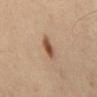  biopsy_status: not biopsied; imaged during a skin examination
  image:
    source: total-body photography crop
    field_of_view_mm: 15
  automated_metrics:
    area_mm2_approx: 4.5
    eccentricity: 0.85
    shape_asymmetry: 0.25
    cielab_L: 50
    cielab_a: 19
    cielab_b: 31
    vs_skin_contrast_norm: 9.5
    nevus_likeness_0_100: 100
  patient:
    sex: male
    age_approx: 55
  lesion_size:
    long_diameter_mm_approx: 3.0
  site: abdomen